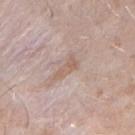Findings:
– notes · imaged on a skin check; not biopsied
– patient · male, aged around 30
– image · ~15 mm crop, total-body skin-cancer survey
– location · the right forearm
– lighting · white-light
– lesion diameter · about 3.5 mm